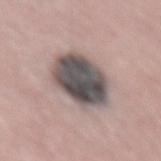{"biopsy_status": "not biopsied; imaged during a skin examination", "patient": {"sex": "male", "age_approx": 55}, "image": {"source": "total-body photography crop", "field_of_view_mm": 15}, "lighting": "white-light", "lesion_size": {"long_diameter_mm_approx": 6.0}, "automated_metrics": {"shape_asymmetry": 0.1, "cielab_L": 48, "cielab_a": 9, "cielab_b": 14, "vs_skin_darker_L": 19.0, "vs_skin_contrast_norm": 13.0, "nevus_likeness_0_100": 15, "lesion_detection_confidence_0_100": 95}}